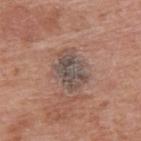follow-up: no biopsy performed (imaged during a skin exam)
location: the right upper arm
imaging modality: 15 mm crop, total-body photography
tile lighting: white-light
size: ~4.5 mm (longest diameter)
subject: male, aged approximately 60
automated metrics: an area of roughly 11 mm², an outline eccentricity of about 0.75 (0 = round, 1 = elongated), and two-axis asymmetry of about 0.25; a lesion–skin lightness drop of about 10 and a normalized border contrast of about 8.5